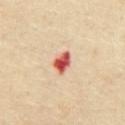Q: Is there a histopathology result?
A: imaged on a skin check; not biopsied
Q: Lesion location?
A: the chest
Q: What kind of image is this?
A: ~15 mm tile from a whole-body skin photo
Q: What are the patient's age and sex?
A: female, about 70 years old